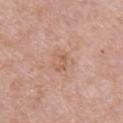Part of a total-body skin-imaging series; this lesion was reviewed on a skin check and was not flagged for biopsy.
A female subject aged around 70.
From the right upper arm.
Cropped from a whole-body photographic skin survey; the tile spans about 15 mm.
Captured under white-light illumination.